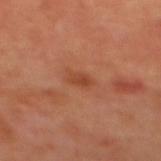Clinical impression: Imaged during a routine full-body skin examination; the lesion was not biopsied and no histopathology is available. Acquisition and patient details: Cropped from a total-body skin-imaging series; the visible field is about 15 mm. The subject is female. Captured under cross-polarized illumination. About 2 mm across. From the upper back.